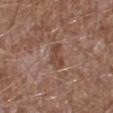  biopsy_status: not biopsied; imaged during a skin examination
  lesion_size:
    long_diameter_mm_approx: 3.0
  lighting: white-light
  patient:
    sex: male
    age_approx: 45
  image:
    source: total-body photography crop
    field_of_view_mm: 15
  site: right lower leg
  automated_metrics:
    border_irregularity_0_10: 3.5
    color_variation_0_10: 2.0
    peripheral_color_asymmetry: 1.0